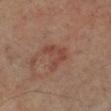• follow-up: no biopsy performed (imaged during a skin exam)
• acquisition: ~15 mm tile from a whole-body skin photo
• diameter: about 3.5 mm
• body site: the left leg
• automated lesion analysis: a lesion color around L≈43 a*≈22 b*≈26 in CIELAB and a lesion–skin lightness drop of about 6
• subject: male, aged around 50